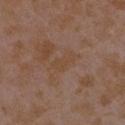Recorded during total-body skin imaging; not selected for excision or biopsy. Cropped from a total-body skin-imaging series; the visible field is about 15 mm. A female patient aged 33 to 37. From the right upper arm.